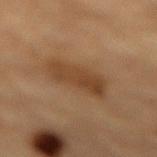Recorded during total-body skin imaging; not selected for excision or biopsy. The subject is a male about 85 years old or older. On the mid back. Cropped from a total-body skin-imaging series; the visible field is about 15 mm.